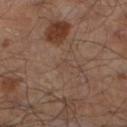This lesion was catalogued during total-body skin photography and was not selected for biopsy. This image is a 15 mm lesion crop taken from a total-body photograph. Located on the left lower leg. The tile uses cross-polarized illumination. A male patient, aged 63–67. The recorded lesion diameter is about 1.5 mm.